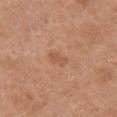Recorded during total-body skin imaging; not selected for excision or biopsy.
The lesion is on the front of the torso.
Longest diameter approximately 2.5 mm.
A female patient about 65 years old.
Cropped from a whole-body photographic skin survey; the tile spans about 15 mm.